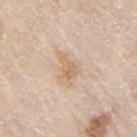workup: no biopsy performed (imaged during a skin exam) | size: ~3.5 mm (longest diameter) | anatomic site: the right upper arm | automated lesion analysis: a border-irregularity index near 6/10, a within-lesion color-variation index near 2.5/10, and radial color variation of about 1 | patient: male, approximately 80 years of age | acquisition: total-body-photography crop, ~15 mm field of view.